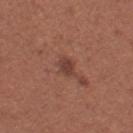Impression: The lesion was tiled from a total-body skin photograph and was not biopsied. Image and clinical context: Measured at roughly 2.5 mm in maximum diameter. The subject is a female roughly 35 years of age. Imaged with white-light lighting. A 15 mm close-up tile from a total-body photography series done for melanoma screening. On the right thigh.